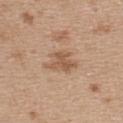Findings:
• workup · total-body-photography surveillance lesion; no biopsy
• lighting · white-light illumination
• lesion diameter · about 4 mm
• acquisition · 15 mm crop, total-body photography
• site · the back
• subject · female, aged approximately 45